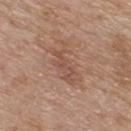Impression:
Imaged during a routine full-body skin examination; the lesion was not biopsied and no histopathology is available.
Acquisition and patient details:
Captured under white-light illumination. A 15 mm crop from a total-body photograph taken for skin-cancer surveillance. A male patient, about 75 years old. The lesion is on the upper back. Measured at roughly 5.5 mm in maximum diameter.